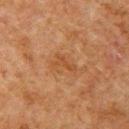Imaged during a routine full-body skin examination; the lesion was not biopsied and no histopathology is available.
A region of skin cropped from a whole-body photographic capture, roughly 15 mm wide.
From the left upper arm.
Measured at roughly 3 mm in maximum diameter.
A male subject, approximately 60 years of age.